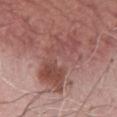The lesion was tiled from a total-body skin photograph and was not biopsied.
Automated tile analysis of the lesion measured a lesion color around L≈48 a*≈24 b*≈24 in CIELAB. It also reported an automated nevus-likeness rating near 0 out of 100 and a detector confidence of about 100 out of 100 that the crop contains a lesion.
Captured under white-light illumination.
On the chest.
A 15 mm crop from a total-body photograph taken for skin-cancer surveillance.
Measured at roughly 8 mm in maximum diameter.
A male subject approximately 60 years of age.